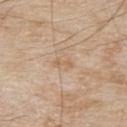{"biopsy_status": "not biopsied; imaged during a skin examination", "patient": {"sex": "male", "age_approx": 80}, "lighting": "white-light", "automated_metrics": {"area_mm2_approx": 2.5, "border_irregularity_0_10": 4.0, "color_variation_0_10": 0.0, "peripheral_color_asymmetry": 0.0}, "site": "upper back", "image": {"source": "total-body photography crop", "field_of_view_mm": 15}}